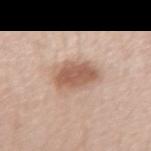Clinical impression: Imaged during a routine full-body skin examination; the lesion was not biopsied and no histopathology is available. Acquisition and patient details: From the left forearm. A female subject, aged around 40. Imaged with white-light lighting. A lesion tile, about 15 mm wide, cut from a 3D total-body photograph.